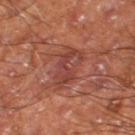Assessment: The lesion was tiled from a total-body skin photograph and was not biopsied. Context: A close-up tile cropped from a whole-body skin photograph, about 15 mm across. A male subject aged approximately 60. The lesion is located on the right thigh.